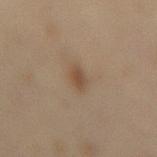image=~15 mm crop, total-body skin-cancer survey; tile lighting=cross-polarized illumination; patient=female, aged approximately 60; size=~2.5 mm (longest diameter); body site=the back.